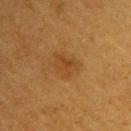Impression:
The lesion was photographed on a routine skin check and not biopsied; there is no pathology result.
Background:
The lesion's longest dimension is about 3.5 mm. The lesion is on the right upper arm. A close-up tile cropped from a whole-body skin photograph, about 15 mm across. A female subject aged around 40. The tile uses cross-polarized illumination.